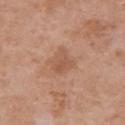| key | value |
|---|---|
| patient | female, roughly 50 years of age |
| body site | the chest |
| image | ~15 mm tile from a whole-body skin photo |